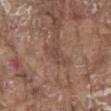The lesion was photographed on a routine skin check and not biopsied; there is no pathology result. The subject is a male about 80 years old. The lesion's longest dimension is about 2.5 mm. Automated tile analysis of the lesion measured a lesion area of about 2 mm², an eccentricity of roughly 0.9, and a symmetry-axis asymmetry near 0.45. And it measured about 7 CIELAB-L* units darker than the surrounding skin. And it measured a border-irregularity index near 4.5/10, a color-variation rating of about 0/10, and peripheral color asymmetry of about 0. The analysis additionally found an automated nevus-likeness rating near 0 out of 100 and a lesion-detection confidence of about 85/100. From the chest. Cropped from a total-body skin-imaging series; the visible field is about 15 mm. The tile uses white-light illumination.